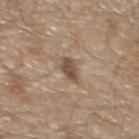follow-up=imaged on a skin check; not biopsied | subject=male, aged approximately 70 | location=the chest | image source=~15 mm tile from a whole-body skin photo.